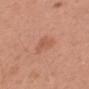Context: A 15 mm close-up extracted from a 3D total-body photography capture. A female patient, aged around 45. Captured under white-light illumination. The lesion is located on the right forearm.